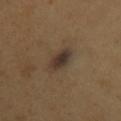biopsy status: imaged on a skin check; not biopsied
image source: ~15 mm tile from a whole-body skin photo
automated lesion analysis: a footprint of about 7.5 mm² and an outline eccentricity of about 0.85 (0 = round, 1 = elongated); an average lesion color of about L≈35 a*≈12 b*≈23 (CIELAB) and about 9 CIELAB-L* units darker than the surrounding skin
location: the left upper arm
subject: female, aged around 60
illumination: cross-polarized illumination
lesion diameter: ≈4.5 mm